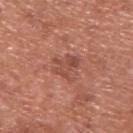Impression: The lesion was photographed on a routine skin check and not biopsied; there is no pathology result. Image and clinical context: Located on the upper back. The subject is a male about 75 years old. Approximately 3.5 mm at its widest. Cropped from a whole-body photographic skin survey; the tile spans about 15 mm. This is a white-light tile. An algorithmic analysis of the crop reported roughly 8 lightness units darker than nearby skin. It also reported a border-irregularity index near 7/10, internal color variation of about 3 on a 0–10 scale, and radial color variation of about 1.